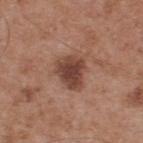biopsy status: imaged on a skin check; not biopsied
patient: male, approximately 55 years of age
site: the upper back
acquisition: ~15 mm tile from a whole-body skin photo